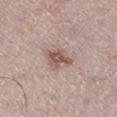Clinical summary:
The subject is a male aged 68–72. The lesion-visualizer software estimated a lesion area of about 6 mm², a shape eccentricity near 0.8, and a shape-asymmetry score of about 0.35 (0 = symmetric). The software also gave a mean CIELAB color near L≈54 a*≈17 b*≈24, about 12 CIELAB-L* units darker than the surrounding skin, and a normalized border contrast of about 8. It also reported radial color variation of about 1. The lesion's longest dimension is about 4 mm. A close-up tile cropped from a whole-body skin photograph, about 15 mm across. On the left lower leg.